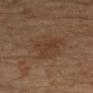Findings:
* site — the leg
* subject — male, aged 28 to 32
* acquisition — ~15 mm tile from a whole-body skin photo